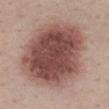Case summary:
* follow-up — imaged on a skin check; not biopsied
* patient — male, in their mid-20s
* image source — ~15 mm crop, total-body skin-cancer survey
* body site — the mid back